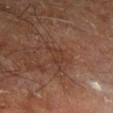Part of a total-body skin-imaging series; this lesion was reviewed on a skin check and was not flagged for biopsy.
A male subject aged approximately 60.
Captured under cross-polarized illumination.
A lesion tile, about 15 mm wide, cut from a 3D total-body photograph.
Automated image analysis of the tile measured an eccentricity of roughly 0.75 and two-axis asymmetry of about 0.45. The analysis additionally found an automated nevus-likeness rating near 0 out of 100 and a detector confidence of about 95 out of 100 that the crop contains a lesion.
The lesion is located on the right leg.